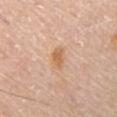follow-up=catalogued during a skin exam; not biopsied | subject=male, roughly 70 years of age | acquisition=total-body-photography crop, ~15 mm field of view | lesion diameter=~3 mm (longest diameter) | tile lighting=white-light illumination | site=the chest.